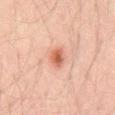Recorded during total-body skin imaging; not selected for excision or biopsy. Imaged with cross-polarized lighting. A male patient aged around 30. The lesion is located on the abdomen. Cropped from a total-body skin-imaging series; the visible field is about 15 mm.